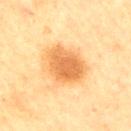{
  "automated_metrics": {
    "cielab_L": 55,
    "cielab_a": 21,
    "cielab_b": 40,
    "vs_skin_darker_L": 11.0,
    "nevus_likeness_0_100": 100,
    "lesion_detection_confidence_0_100": 100
  },
  "image": {
    "source": "total-body photography crop",
    "field_of_view_mm": 15
  },
  "site": "left upper arm",
  "lesion_size": {
    "long_diameter_mm_approx": 5.0
  },
  "patient": {
    "sex": "male",
    "age_approx": 65
  },
  "lighting": "cross-polarized"
}